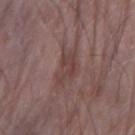workup: no biopsy performed (imaged during a skin exam)
lighting: white-light illumination
imaging modality: 15 mm crop, total-body photography
body site: the right forearm
patient: male, aged 63–67
size: about 5 mm